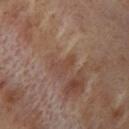notes — imaged on a skin check; not biopsied.